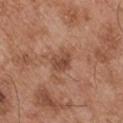Acquisition and patient details: The lesion-visualizer software estimated a border-irregularity rating of about 2.5/10 and radial color variation of about 1. It also reported a classifier nevus-likeness of about 5/100 and lesion-presence confidence of about 100/100. On the left upper arm. Longest diameter approximately 3 mm. A roughly 15 mm field-of-view crop from a total-body skin photograph. The subject is a male about 55 years old.